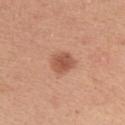Imaged during a routine full-body skin examination; the lesion was not biopsied and no histopathology is available. Measured at roughly 3 mm in maximum diameter. A roughly 15 mm field-of-view crop from a total-body skin photograph. A female subject, aged approximately 45. The tile uses white-light illumination. The total-body-photography lesion software estimated a lesion area of about 6 mm² and an outline eccentricity of about 0.5 (0 = round, 1 = elongated). The analysis additionally found a lesion color around L≈54 a*≈25 b*≈32 in CIELAB and a normalized border contrast of about 7.5. And it measured a peripheral color-asymmetry measure near 1. The software also gave a classifier nevus-likeness of about 95/100 and lesion-presence confidence of about 100/100. From the left upper arm.